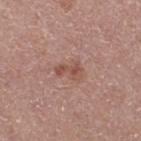notes: total-body-photography surveillance lesion; no biopsy | acquisition: 15 mm crop, total-body photography | diameter: ≈3 mm | tile lighting: white-light illumination | patient: female, aged 48–52 | anatomic site: the right thigh.